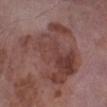The lesion was tiled from a total-body skin photograph and was not biopsied.
This image is a 15 mm lesion crop taken from a total-body photograph.
On the left lower leg.
Captured under white-light illumination.
About 8.5 mm across.
A male patient, aged 73–77.
Automated tile analysis of the lesion measured a footprint of about 40 mm², an eccentricity of roughly 0.5, and a symmetry-axis asymmetry near 0.4. The analysis additionally found an average lesion color of about L≈41 a*≈21 b*≈22 (CIELAB) and a normalized border contrast of about 7.5.